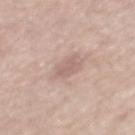{"biopsy_status": "not biopsied; imaged during a skin examination", "image": {"source": "total-body photography crop", "field_of_view_mm": 15}, "site": "mid back", "patient": {"sex": "male", "age_approx": 55}}